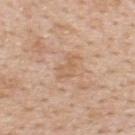Notes:
• follow-up: total-body-photography surveillance lesion; no biopsy
• acquisition: 15 mm crop, total-body photography
• location: the upper back
• illumination: white-light
• automated metrics: a border-irregularity rating of about 4.5/10 and a color-variation rating of about 2/10
• patient: male, aged 48–52